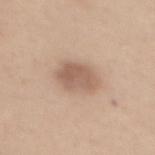| field | value |
|---|---|
| notes | catalogued during a skin exam; not biopsied |
| illumination | white-light illumination |
| image source | ~15 mm tile from a whole-body skin photo |
| subject | female, aged 43–47 |
| automated lesion analysis | an outline eccentricity of about 0.65 (0 = round, 1 = elongated); an average lesion color of about L≈59 a*≈17 b*≈28 (CIELAB) and roughly 11 lightness units darker than nearby skin; a color-variation rating of about 3/10 and a peripheral color-asymmetry measure near 1 |
| size | about 4 mm |
| body site | the upper back |